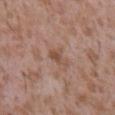Case summary:
• illumination — white-light illumination
• anatomic site — the mid back
• lesion size — about 3 mm
• patient — male, aged 43 to 47
• imaging modality — ~15 mm crop, total-body skin-cancer survey